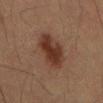notes: imaged on a skin check; not biopsied
TBP lesion metrics: an outline eccentricity of about 0.85 (0 = round, 1 = elongated) and a symmetry-axis asymmetry near 0.15
illumination: cross-polarized
anatomic site: the mid back
patient: male, aged 53–57
lesion size: ≈6 mm
imaging modality: ~15 mm tile from a whole-body skin photo